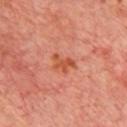This lesion was catalogued during total-body skin photography and was not selected for biopsy. The lesion is on the chest. A region of skin cropped from a whole-body photographic capture, roughly 15 mm wide. Captured under cross-polarized illumination. The lesion-visualizer software estimated a footprint of about 4 mm² and a shape-asymmetry score of about 0.5 (0 = symmetric). And it measured about 9 CIELAB-L* units darker than the surrounding skin and a normalized border contrast of about 7.5. And it measured border irregularity of about 6 on a 0–10 scale, a color-variation rating of about 1/10, and radial color variation of about 0. The software also gave a classifier nevus-likeness of about 0/100. The subject is a male approximately 65 years of age. Approximately 3 mm at its widest.